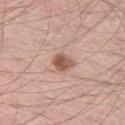Clinical impression: Recorded during total-body skin imaging; not selected for excision or biopsy. Background: The subject is a male in their mid- to late 50s. The lesion is on the left thigh. This is a white-light tile. Approximately 2.5 mm at its widest. An algorithmic analysis of the crop reported a footprint of about 4.5 mm², an outline eccentricity of about 0.55 (0 = round, 1 = elongated), and a symmetry-axis asymmetry near 0.2. The analysis additionally found a lesion color around L≈55 a*≈22 b*≈27 in CIELAB, about 13 CIELAB-L* units darker than the surrounding skin, and a normalized lesion–skin contrast near 9. The software also gave a border-irregularity rating of about 2/10 and a color-variation rating of about 4/10. The analysis additionally found a classifier nevus-likeness of about 90/100 and a lesion-detection confidence of about 100/100. A 15 mm close-up extracted from a 3D total-body photography capture.